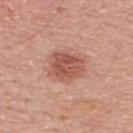Image and clinical context: A male subject, approximately 65 years of age. Captured under white-light illumination. An algorithmic analysis of the crop reported an eccentricity of roughly 0.6 and a symmetry-axis asymmetry near 0.15. The software also gave border irregularity of about 1.5 on a 0–10 scale, internal color variation of about 4 on a 0–10 scale, and radial color variation of about 1.5. Located on the upper back. This image is a 15 mm lesion crop taken from a total-body photograph. Approximately 5 mm at its widest.